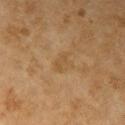Findings:
• follow-up: imaged on a skin check; not biopsied
• site: the right upper arm
• lesion size: ≈2.5 mm
• patient: female, roughly 60 years of age
• tile lighting: cross-polarized illumination
• image source: ~15 mm tile from a whole-body skin photo